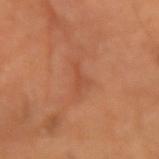Impression:
The lesion was photographed on a routine skin check and not biopsied; there is no pathology result.
Acquisition and patient details:
Automated tile analysis of the lesion measured roughly 6 lightness units darker than nearby skin and a lesion-to-skin contrast of about 4.5 (normalized; higher = more distinct). It also reported a border-irregularity rating of about 7/10. The software also gave a nevus-likeness score of about 0/100 and lesion-presence confidence of about 95/100. About 3.5 mm across. The patient is a female about 70 years old. This is a cross-polarized tile. The lesion is located on the left upper arm. A 15 mm close-up tile from a total-body photography series done for melanoma screening.